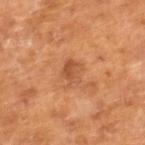Notes:
* notes — total-body-photography surveillance lesion; no biopsy
* automated lesion analysis — a lesion color around L≈52 a*≈25 b*≈38 in CIELAB and a lesion–skin lightness drop of about 8; a nevus-likeness score of about 0/100 and a detector confidence of about 100 out of 100 that the crop contains a lesion
* tile lighting — cross-polarized illumination
* diameter — about 3 mm
* image source — total-body-photography crop, ~15 mm field of view
* patient — male, aged 63 to 67